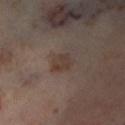Imaged during a routine full-body skin examination; the lesion was not biopsied and no histopathology is available. A 15 mm crop from a total-body photograph taken for skin-cancer surveillance. The lesion is located on the left lower leg. The subject is a female in their mid-50s. Automated image analysis of the tile measured a lesion color around L≈38 a*≈14 b*≈21 in CIELAB, a lesion–skin lightness drop of about 6, and a normalized border contrast of about 6.5. About 3 mm across. Imaged with cross-polarized lighting.